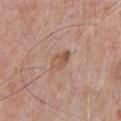follow-up: imaged on a skin check; not biopsied
acquisition: ~15 mm tile from a whole-body skin photo
image-analysis metrics: a footprint of about 4.5 mm², an eccentricity of roughly 0.85, and a shape-asymmetry score of about 0.3 (0 = symmetric); a lesion color around L≈54 a*≈20 b*≈30 in CIELAB, roughly 8 lightness units darker than nearby skin, and a normalized lesion–skin contrast near 7
size: about 3 mm
patient: male, about 70 years old
tile lighting: white-light illumination
anatomic site: the chest